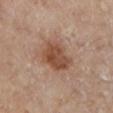Impression: This lesion was catalogued during total-body skin photography and was not selected for biopsy. Image and clinical context: A roughly 15 mm field-of-view crop from a total-body skin photograph. The subject is a female aged 58–62. Located on the left lower leg. Captured under cross-polarized illumination. Measured at roughly 4 mm in maximum diameter.